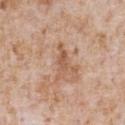Clinical impression: Captured during whole-body skin photography for melanoma surveillance; the lesion was not biopsied. Background: Longest diameter approximately 4.5 mm. A close-up tile cropped from a whole-body skin photograph, about 15 mm across. The total-body-photography lesion software estimated roughly 9 lightness units darker than nearby skin and a normalized border contrast of about 6.5. Imaged with white-light lighting. The patient is a male roughly 65 years of age. From the front of the torso.